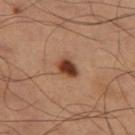<record>
  <biopsy_status>not biopsied; imaged during a skin examination</biopsy_status>
  <site>left thigh</site>
  <lighting>cross-polarized</lighting>
  <lesion_size>
    <long_diameter_mm_approx>3.0</long_diameter_mm_approx>
  </lesion_size>
  <image>
    <source>total-body photography crop</source>
    <field_of_view_mm>15</field_of_view_mm>
  </image>
  <patient>
    <sex>male</sex>
    <age_approx>60</age_approx>
  </patient>
  <automated_metrics>
    <cielab_L>40</cielab_L>
    <cielab_a>23</cielab_a>
    <cielab_b>31</cielab_b>
    <vs_skin_darker_L>16.0</vs_skin_darker_L>
    <vs_skin_contrast_norm>12.5</vs_skin_contrast_norm>
    <color_variation_0_10>3.5</color_variation_0_10>
    <peripheral_color_asymmetry>1.0</peripheral_color_asymmetry>
  </automated_metrics>
</record>